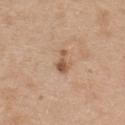Recorded during total-body skin imaging; not selected for excision or biopsy. A female patient aged 43–47. The lesion is on the upper back. An algorithmic analysis of the crop reported an average lesion color of about L≈55 a*≈19 b*≈32 (CIELAB), a lesion–skin lightness drop of about 11, and a normalized border contrast of about 7.5. The analysis additionally found a border-irregularity index near 5/10 and a within-lesion color-variation index near 1.5/10. It also reported a classifier nevus-likeness of about 45/100 and a detector confidence of about 100 out of 100 that the crop contains a lesion. This image is a 15 mm lesion crop taken from a total-body photograph.